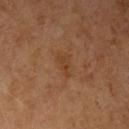The lesion was tiled from a total-body skin photograph and was not biopsied. A 15 mm close-up tile from a total-body photography series done for melanoma screening. The subject is a female in their 60s. On the left upper arm. Measured at roughly 3 mm in maximum diameter. Automated image analysis of the tile measured a footprint of about 4 mm², a shape eccentricity near 0.8, and a symmetry-axis asymmetry near 0.4. It also reported a mean CIELAB color near L≈35 a*≈19 b*≈30, roughly 5 lightness units darker than nearby skin, and a normalized border contrast of about 5.5. And it measured internal color variation of about 1 on a 0–10 scale and a peripheral color-asymmetry measure near 0.5. This is a cross-polarized tile.